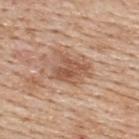<lesion>
  <biopsy_status>not biopsied; imaged during a skin examination</biopsy_status>
  <image>
    <source>total-body photography crop</source>
    <field_of_view_mm>15</field_of_view_mm>
  </image>
  <patient>
    <sex>male</sex>
    <age_approx>60</age_approx>
  </patient>
  <site>upper back</site>
  <automated_metrics>
    <area_mm2_approx>12.0</area_mm2_approx>
    <eccentricity>0.7</eccentricity>
    <cielab_L>55</cielab_L>
    <cielab_a>20</cielab_a>
    <cielab_b>31</cielab_b>
    <vs_skin_darker_L>10.0</vs_skin_darker_L>
  </automated_metrics>
  <lesion_size>
    <long_diameter_mm_approx>4.5</long_diameter_mm_approx>
  </lesion_size>
</lesion>